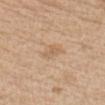follow-up: imaged on a skin check; not biopsied | image: ~15 mm tile from a whole-body skin photo | illumination: white-light | body site: the front of the torso | lesion diameter: ~3 mm (longest diameter) | automated metrics: a nevus-likeness score of about 0/100 and lesion-presence confidence of about 100/100 | patient: male, in their mid-40s.